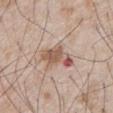workup = total-body-photography surveillance lesion; no biopsy
image-analysis metrics = a lesion area of about 9 mm², a shape eccentricity near 0.65, and two-axis asymmetry of about 0.35; a border-irregularity rating of about 4/10, a within-lesion color-variation index near 7/10, and radial color variation of about 2; an automated nevus-likeness rating near 5 out of 100 and lesion-presence confidence of about 100/100
lighting = white-light
subject = male, aged approximately 65
image = ~15 mm tile from a whole-body skin photo
site = the chest